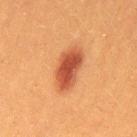Q: Was a biopsy performed?
A: total-body-photography surveillance lesion; no biopsy